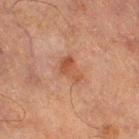<tbp_lesion>
  <biopsy_status>not biopsied; imaged during a skin examination</biopsy_status>
  <image>
    <source>total-body photography crop</source>
    <field_of_view_mm>15</field_of_view_mm>
  </image>
  <lighting>cross-polarized</lighting>
  <site>left thigh</site>
  <patient>
    <sex>male</sex>
    <age_approx>65</age_approx>
  </patient>
</tbp_lesion>